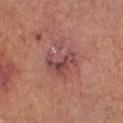Impression:
Recorded during total-body skin imaging; not selected for excision or biopsy.
Context:
A 15 mm crop from a total-body photograph taken for skin-cancer surveillance. A female patient in their mid- to late 60s. About 4.5 mm across. The tile uses white-light illumination. From the front of the torso.